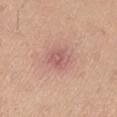Recorded during total-body skin imaging; not selected for excision or biopsy. This is a white-light tile. A female subject, in their 40s. Approximately 3 mm at its widest. A close-up tile cropped from a whole-body skin photograph, about 15 mm across. The lesion is on the left thigh.